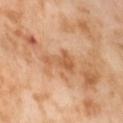Assessment: The lesion was tiled from a total-body skin photograph and was not biopsied. Context: A female patient, aged around 55. Captured under cross-polarized illumination. Longest diameter approximately 4 mm. On the left thigh. Cropped from a total-body skin-imaging series; the visible field is about 15 mm.